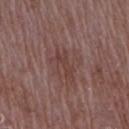No biopsy was performed on this lesion — it was imaged during a full skin examination and was not determined to be concerning.
Automated tile analysis of the lesion measured an area of roughly 5.5 mm², an outline eccentricity of about 0.9 (0 = round, 1 = elongated), and a symmetry-axis asymmetry near 0.45. The analysis additionally found a lesion color around L≈40 a*≈19 b*≈21 in CIELAB, a lesion–skin lightness drop of about 6, and a normalized lesion–skin contrast near 5.5. It also reported border irregularity of about 6.5 on a 0–10 scale. The analysis additionally found a classifier nevus-likeness of about 0/100 and lesion-presence confidence of about 90/100.
From the right upper arm.
A lesion tile, about 15 mm wide, cut from a 3D total-body photograph.
The patient is a female in their 70s.
Approximately 4 mm at its widest.